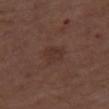<lesion>
<biopsy_status>not biopsied; imaged during a skin examination</biopsy_status>
<automated_metrics>
  <area_mm2_approx>6.0</area_mm2_approx>
  <eccentricity>0.65</eccentricity>
  <shape_asymmetry>0.25</shape_asymmetry>
  <vs_skin_darker_L>5.0</vs_skin_darker_L>
  <vs_skin_contrast_norm>5.0</vs_skin_contrast_norm>
  <border_irregularity_0_10>2.5</border_irregularity_0_10>
  <peripheral_color_asymmetry>0.5</peripheral_color_asymmetry>
</automated_metrics>
<image>
  <source>total-body photography crop</source>
  <field_of_view_mm>15</field_of_view_mm>
</image>
<lesion_size>
  <long_diameter_mm_approx>3.0</long_diameter_mm_approx>
</lesion_size>
<lighting>white-light</lighting>
<site>leg</site>
<patient>
  <sex>male</sex>
  <age_approx>75</age_approx>
</patient>
</lesion>